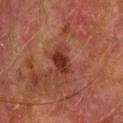Imaged during a routine full-body skin examination; the lesion was not biopsied and no histopathology is available. A 15 mm close-up tile from a total-body photography series done for melanoma screening. The lesion's longest dimension is about 3.5 mm. From the left forearm. Automated image analysis of the tile measured a lesion color around L≈28 a*≈23 b*≈26 in CIELAB, about 9 CIELAB-L* units darker than the surrounding skin, and a normalized lesion–skin contrast near 9. And it measured a border-irregularity rating of about 3/10, internal color variation of about 3 on a 0–10 scale, and a peripheral color-asymmetry measure near 1. The analysis additionally found an automated nevus-likeness rating near 55 out of 100 and a detector confidence of about 100 out of 100 that the crop contains a lesion. Imaged with cross-polarized lighting. The patient is a male about 75 years old.